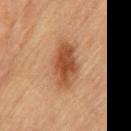Imaged during a routine full-body skin examination; the lesion was not biopsied and no histopathology is available. From the chest. Captured under cross-polarized illumination. A 15 mm close-up tile from a total-body photography series done for melanoma screening. A male subject aged approximately 85 or older. The recorded lesion diameter is about 5.5 mm.